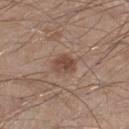Q: Was this lesion biopsied?
A: total-body-photography surveillance lesion; no biopsy
Q: What is the lesion's diameter?
A: ≈3 mm
Q: How was this image acquired?
A: ~15 mm tile from a whole-body skin photo
Q: What are the patient's age and sex?
A: male, aged 28–32
Q: What did automated image analysis measure?
A: an average lesion color of about L≈46 a*≈18 b*≈26 (CIELAB) and about 10 CIELAB-L* units darker than the surrounding skin; a border-irregularity rating of about 1.5/10 and peripheral color asymmetry of about 1
Q: Lesion location?
A: the leg
Q: What lighting was used for the tile?
A: white-light illumination